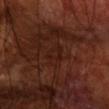The lesion was tiled from a total-body skin photograph and was not biopsied. A male subject approximately 70 years of age. On the arm. Longest diameter approximately 4 mm. A close-up tile cropped from a whole-body skin photograph, about 15 mm across.